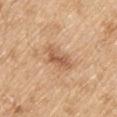Imaged with white-light lighting. The lesion is located on the upper back. The lesion-visualizer software estimated a footprint of about 6.5 mm² and two-axis asymmetry of about 0.3. The software also gave a nevus-likeness score of about 0/100. The subject is a male approximately 70 years of age. A region of skin cropped from a whole-body photographic capture, roughly 15 mm wide.